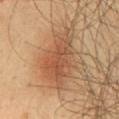Background: The tile uses cross-polarized illumination. A roughly 15 mm field-of-view crop from a total-body skin photograph. A male subject approximately 50 years of age. The lesion's longest dimension is about 8 mm. From the back.